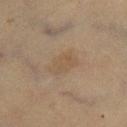Image and clinical context: A female patient aged approximately 55. The lesion-visualizer software estimated an average lesion color of about L≈43 a*≈12 b*≈26 (CIELAB), a lesion–skin lightness drop of about 5, and a normalized lesion–skin contrast near 4.5. The software also gave an automated nevus-likeness rating near 0 out of 100 and lesion-presence confidence of about 100/100. A lesion tile, about 15 mm wide, cut from a 3D total-body photograph. The lesion's longest dimension is about 4 mm. Captured under cross-polarized illumination. The lesion is on the right lower leg.